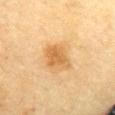{"biopsy_status": "not biopsied; imaged during a skin examination", "lesion_size": {"long_diameter_mm_approx": 3.5}, "site": "chest", "patient": {"sex": "female", "age_approx": 55}, "image": {"source": "total-body photography crop", "field_of_view_mm": 15}}